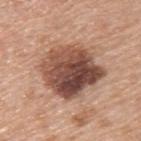Measured at roughly 7.5 mm in maximum diameter. Automated image analysis of the tile measured roughly 17 lightness units darker than nearby skin. And it measured a border-irregularity rating of about 2/10, internal color variation of about 9.5 on a 0–10 scale, and a peripheral color-asymmetry measure near 3.5. A 15 mm close-up tile from a total-body photography series done for melanoma screening. A male subject, about 50 years old. On the back. On excision, pathology confirmed an invasive melanoma, superficial spreading type; Breslow depth 0.4 mm, mitotic rate <1/mm².